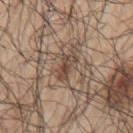Imaged during a routine full-body skin examination; the lesion was not biopsied and no histopathology is available. Cropped from a whole-body photographic skin survey; the tile spans about 15 mm. Approximately 3 mm at its widest. The lesion is located on the left upper arm. A male subject, aged around 70. Captured under white-light illumination.